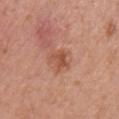Q: Was a biopsy performed?
A: imaged on a skin check; not biopsied
Q: Lesion location?
A: the right upper arm
Q: What is the lesion's diameter?
A: ~3 mm (longest diameter)
Q: Automated lesion metrics?
A: roughly 9 lightness units darker than nearby skin and a lesion-to-skin contrast of about 7 (normalized; higher = more distinct); a within-lesion color-variation index near 2.5/10 and radial color variation of about 1
Q: Illumination type?
A: white-light
Q: Patient demographics?
A: female, aged 48–52
Q: How was this image acquired?
A: total-body-photography crop, ~15 mm field of view